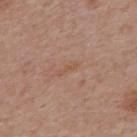image source = ~15 mm crop, total-body skin-cancer survey
size = about 2.5 mm
subject = male, aged approximately 60
location = the upper back